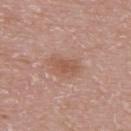Case summary:
– workup: catalogued during a skin exam; not biopsied
– anatomic site: the upper back
– TBP lesion metrics: a symmetry-axis asymmetry near 0.3; a mean CIELAB color near L≈54 a*≈22 b*≈29 and about 8 CIELAB-L* units darker than the surrounding skin; internal color variation of about 1.5 on a 0–10 scale and radial color variation of about 0.5
– imaging modality: 15 mm crop, total-body photography
– illumination: white-light
– lesion diameter: about 3 mm
– subject: female, aged 28–32